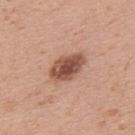The lesion was tiled from a total-body skin photograph and was not biopsied. On the upper back. A close-up tile cropped from a whole-body skin photograph, about 15 mm across. About 4.5 mm across. Automated image analysis of the tile measured an automated nevus-likeness rating near 90 out of 100 and lesion-presence confidence of about 100/100. This is a white-light tile. A male patient, roughly 30 years of age.